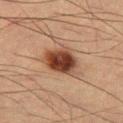Imaged during a routine full-body skin examination; the lesion was not biopsied and no histopathology is available. The recorded lesion diameter is about 4.5 mm. On the right thigh. Imaged with cross-polarized lighting. An algorithmic analysis of the crop reported border irregularity of about 2.5 on a 0–10 scale and a color-variation rating of about 7/10. The analysis additionally found a nevus-likeness score of about 100/100 and a lesion-detection confidence of about 100/100. Cropped from a total-body skin-imaging series; the visible field is about 15 mm. The subject is a male about 55 years old.